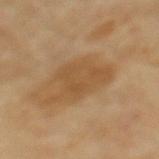biopsy status: imaged on a skin check; not biopsied